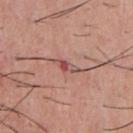image = 15 mm crop, total-body photography; image-analysis metrics = an automated nevus-likeness rating near 0 out of 100 and a detector confidence of about 95 out of 100 that the crop contains a lesion; anatomic site = the front of the torso; diameter = ~3 mm (longest diameter); patient = male, roughly 55 years of age; lighting = white-light illumination.